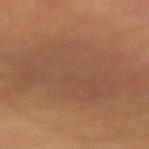Imaged during a routine full-body skin examination; the lesion was not biopsied and no histopathology is available.
A female subject, about 70 years old.
The lesion is on the left forearm.
Longest diameter approximately 12 mm.
An algorithmic analysis of the crop reported a lesion area of about 60 mm², an outline eccentricity of about 0.85 (0 = round, 1 = elongated), and a symmetry-axis asymmetry near 0.3. It also reported a lesion color around L≈39 a*≈17 b*≈26 in CIELAB and a normalized border contrast of about 4.5. And it measured a border-irregularity index near 6.5/10, a within-lesion color-variation index near 2.5/10, and radial color variation of about 0.5.
A lesion tile, about 15 mm wide, cut from a 3D total-body photograph.
This is a cross-polarized tile.